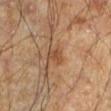The lesion was photographed on a routine skin check and not biopsied; there is no pathology result.
A male subject aged 58–62.
From the left lower leg.
A close-up tile cropped from a whole-body skin photograph, about 15 mm across.